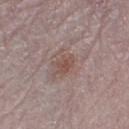Impression:
This lesion was catalogued during total-body skin photography and was not selected for biopsy.
Acquisition and patient details:
About 2.5 mm across. A 15 mm close-up extracted from a 3D total-body photography capture. The tile uses white-light illumination. Located on the left lower leg. The patient is a female in their mid-60s. Automated image analysis of the tile measured a border-irregularity index near 4/10, a within-lesion color-variation index near 2/10, and peripheral color asymmetry of about 0.5. The analysis additionally found a lesion-detection confidence of about 100/100.